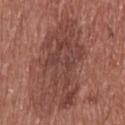<record>
  <biopsy_status>not biopsied; imaged during a skin examination</biopsy_status>
  <lesion_size>
    <long_diameter_mm_approx>10.0</long_diameter_mm_approx>
  </lesion_size>
  <lighting>white-light</lighting>
  <patient>
    <sex>male</sex>
    <age_approx>75</age_approx>
  </patient>
  <site>upper back</site>
  <image>
    <source>total-body photography crop</source>
    <field_of_view_mm>15</field_of_view_mm>
  </image>
  <automated_metrics>
    <nevus_likeness_0_100>0</nevus_likeness_0_100>
    <lesion_detection_confidence_0_100>100</lesion_detection_confidence_0_100>
  </automated_metrics>
</record>